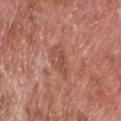Captured during whole-body skin photography for melanoma surveillance; the lesion was not biopsied. A 15 mm crop from a total-body photograph taken for skin-cancer surveillance. A male subject about 65 years old. About 4 mm across. The tile uses white-light illumination. The lesion is on the head or neck.